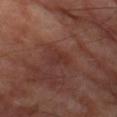workup — catalogued during a skin exam; not biopsied | patient — male, aged 68 to 72 | lesion size — ~3 mm (longest diameter) | body site — the left thigh | image — ~15 mm tile from a whole-body skin photo | TBP lesion metrics — a lesion area of about 4 mm², a shape eccentricity near 0.85, and two-axis asymmetry of about 0.5; a mean CIELAB color near L≈31 a*≈22 b*≈23 and a normalized border contrast of about 5; an automated nevus-likeness rating near 0 out of 100.